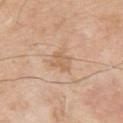notes = no biopsy performed (imaged during a skin exam); anatomic site = the upper back; image = ~15 mm crop, total-body skin-cancer survey; patient = male, aged 78–82.